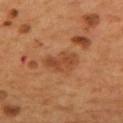Findings:
- patient: male, aged 53 to 57
- anatomic site: the mid back
- image source: total-body-photography crop, ~15 mm field of view